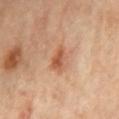{
  "biopsy_status": "not biopsied; imaged during a skin examination",
  "image": {
    "source": "total-body photography crop",
    "field_of_view_mm": 15
  },
  "site": "chest",
  "automated_metrics": {
    "area_mm2_approx": 5.0,
    "eccentricity": 0.85,
    "shape_asymmetry": 0.3,
    "border_irregularity_0_10": 3.0,
    "peripheral_color_asymmetry": 1.0,
    "nevus_likeness_0_100": 75,
    "lesion_detection_confidence_0_100": 100
  },
  "lesion_size": {
    "long_diameter_mm_approx": 3.5
  },
  "lighting": "cross-polarized",
  "patient": {
    "sex": "female",
    "age_approx": 60
  }
}